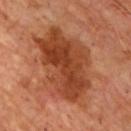{
  "biopsy_status": "not biopsied; imaged during a skin examination",
  "automated_metrics": {
    "cielab_L": 43,
    "cielab_a": 27,
    "cielab_b": 35,
    "vs_skin_contrast_norm": 9.0,
    "nevus_likeness_0_100": 45,
    "lesion_detection_confidence_0_100": 100
  },
  "lesion_size": {
    "long_diameter_mm_approx": 10.0
  },
  "image": {
    "source": "total-body photography crop",
    "field_of_view_mm": 15
  },
  "patient": {
    "sex": "male",
    "age_approx": 65
  },
  "lighting": "cross-polarized"
}